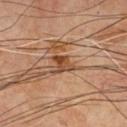Q: Is there a histopathology result?
A: catalogued during a skin exam; not biopsied